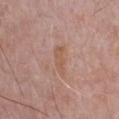workup — catalogued during a skin exam; not biopsied | tile lighting — white-light | automated metrics — a mean CIELAB color near L≈56 a*≈20 b*≈28, a lesion–skin lightness drop of about 6, and a lesion-to-skin contrast of about 5.5 (normalized; higher = more distinct); border irregularity of about 4 on a 0–10 scale and radial color variation of about 0.5 | image — total-body-photography crop, ~15 mm field of view | subject — male, aged 63 to 67 | body site — the chest.